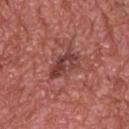| feature | finding |
|---|---|
| notes | catalogued during a skin exam; not biopsied |
| anatomic site | the upper back |
| TBP lesion metrics | a border-irregularity index near 4/10, internal color variation of about 5.5 on a 0–10 scale, and peripheral color asymmetry of about 1.5; a detector confidence of about 100 out of 100 that the crop contains a lesion |
| lighting | white-light illumination |
| imaging modality | ~15 mm crop, total-body skin-cancer survey |
| patient | male, aged 68 to 72 |